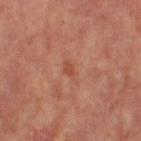Assessment: Part of a total-body skin-imaging series; this lesion was reviewed on a skin check and was not flagged for biopsy. Context: This is a cross-polarized tile. The recorded lesion diameter is about 3 mm. A region of skin cropped from a whole-body photographic capture, roughly 15 mm wide. An algorithmic analysis of the crop reported an area of roughly 3 mm² and two-axis asymmetry of about 0.35. And it measured a lesion color around L≈48 a*≈27 b*≈30 in CIELAB and a normalized lesion–skin contrast near 5. The analysis additionally found border irregularity of about 4 on a 0–10 scale and radial color variation of about 0. It also reported a nevus-likeness score of about 0/100 and lesion-presence confidence of about 100/100. From the left leg. A female subject, approximately 65 years of age.